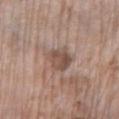Part of a total-body skin-imaging series; this lesion was reviewed on a skin check and was not flagged for biopsy.
A 15 mm close-up extracted from a 3D total-body photography capture.
This is a white-light tile.
The total-body-photography lesion software estimated an area of roughly 7 mm² and an outline eccentricity of about 0.5 (0 = round, 1 = elongated). And it measured a mean CIELAB color near L≈50 a*≈17 b*≈24, about 10 CIELAB-L* units darker than the surrounding skin, and a lesion-to-skin contrast of about 7.5 (normalized; higher = more distinct).
From the left lower leg.
The recorded lesion diameter is about 3 mm.
The patient is a female approximately 75 years of age.